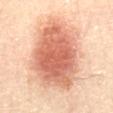No biopsy was performed on this lesion — it was imaged during a full skin examination and was not determined to be concerning.
The subject is a male aged approximately 85 or older.
A lesion tile, about 15 mm wide, cut from a 3D total-body photograph.
The lesion is located on the front of the torso.